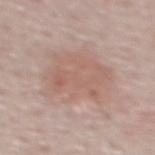Part of a total-body skin-imaging series; this lesion was reviewed on a skin check and was not flagged for biopsy. From the upper back. This is a white-light tile. A male patient aged around 50. This image is a 15 mm lesion crop taken from a total-body photograph. The lesion-visualizer software estimated an average lesion color of about L≈60 a*≈18 b*≈24 (CIELAB) and a lesion–skin lightness drop of about 7. It also reported a classifier nevus-likeness of about 60/100 and a lesion-detection confidence of about 100/100. Longest diameter approximately 8 mm.